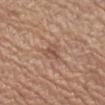Part of a total-body skin-imaging series; this lesion was reviewed on a skin check and was not flagged for biopsy. About 2.5 mm across. A 15 mm close-up tile from a total-body photography series done for melanoma screening. Located on the arm. The patient is a male roughly 65 years of age.